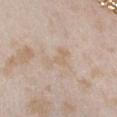workup: no biopsy performed (imaged during a skin exam)
subject: female, aged around 25
imaging modality: 15 mm crop, total-body photography
image-analysis metrics: a lesion–skin lightness drop of about 5
body site: the front of the torso